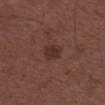Assessment: The lesion was photographed on a routine skin check and not biopsied; there is no pathology result. Context: A male subject, in their 50s. This is a white-light tile. Cropped from a total-body skin-imaging series; the visible field is about 15 mm. The total-body-photography lesion software estimated an average lesion color of about L≈32 a*≈20 b*≈23 (CIELAB), roughly 6 lightness units darker than nearby skin, and a normalized lesion–skin contrast near 6. The analysis additionally found a border-irregularity index near 2.5/10, a color-variation rating of about 2/10, and peripheral color asymmetry of about 0.5.